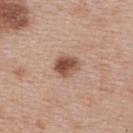Findings:
- follow-up · imaged on a skin check; not biopsied
- lighting · white-light
- TBP lesion metrics · a lesion area of about 6.5 mm², a shape eccentricity near 0.55, and a symmetry-axis asymmetry near 0.2; a nevus-likeness score of about 95/100 and lesion-presence confidence of about 100/100
- location · the upper back
- imaging modality · ~15 mm tile from a whole-body skin photo
- subject · female, about 50 years old
- diameter · about 3 mm